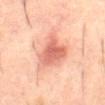Assessment: Recorded during total-body skin imaging; not selected for excision or biopsy. Clinical summary: The lesion's longest dimension is about 4 mm. Imaged with cross-polarized lighting. On the abdomen. A 15 mm close-up extracted from a 3D total-body photography capture. The subject is a male roughly 60 years of age.